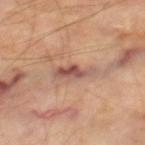Impression: Recorded during total-body skin imaging; not selected for excision or biopsy. Acquisition and patient details: The lesion is located on the right thigh. Cropped from a whole-body photographic skin survey; the tile spans about 15 mm. Imaged with cross-polarized lighting. The subject is a male roughly 70 years of age.